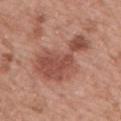Q: Is there a histopathology result?
A: catalogued during a skin exam; not biopsied
Q: Lesion location?
A: the upper back
Q: What is the imaging modality?
A: ~15 mm crop, total-body skin-cancer survey
Q: Lesion size?
A: about 8 mm
Q: Automated lesion metrics?
A: a footprint of about 24 mm² and a shape eccentricity near 0.8; a border-irregularity rating of about 7/10, a color-variation rating of about 4.5/10, and radial color variation of about 1.5
Q: Illumination type?
A: white-light illumination
Q: Who is the patient?
A: male, in their mid-60s